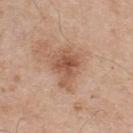Findings:
– workup · catalogued during a skin exam; not biopsied
– patient · male, aged approximately 55
– illumination · white-light illumination
– diameter · ≈5 mm
– site · the back
– image source · total-body-photography crop, ~15 mm field of view